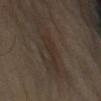This lesion was catalogued during total-body skin photography and was not selected for biopsy. Captured under cross-polarized illumination. Cropped from a whole-body photographic skin survey; the tile spans about 15 mm. A male patient, in their mid-60s. The recorded lesion diameter is about 4.5 mm. The lesion is located on the left upper arm.